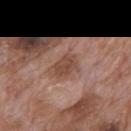{
  "biopsy_status": "not biopsied; imaged during a skin examination",
  "site": "mid back",
  "lighting": "white-light",
  "patient": {
    "sex": "male",
    "age_approx": 70
  },
  "image": {
    "source": "total-body photography crop",
    "field_of_view_mm": 15
  }
}